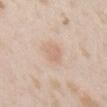Q: Was this lesion biopsied?
A: catalogued during a skin exam; not biopsied
Q: Who is the patient?
A: female, aged approximately 25
Q: How was this image acquired?
A: ~15 mm tile from a whole-body skin photo
Q: Where on the body is the lesion?
A: the chest